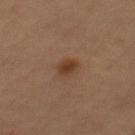Imaged during a routine full-body skin examination; the lesion was not biopsied and no histopathology is available. The total-body-photography lesion software estimated an area of roughly 4 mm² and an outline eccentricity of about 0.75 (0 = round, 1 = elongated). The analysis additionally found a lesion color around L≈34 a*≈17 b*≈27 in CIELAB, about 8 CIELAB-L* units darker than the surrounding skin, and a normalized lesion–skin contrast near 8. It also reported a border-irregularity index near 2.5/10, a color-variation rating of about 3/10, and peripheral color asymmetry of about 1. The analysis additionally found an automated nevus-likeness rating near 100 out of 100 and a lesion-detection confidence of about 100/100. A male patient, aged 63–67. Located on the mid back. A region of skin cropped from a whole-body photographic capture, roughly 15 mm wide.